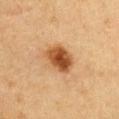This lesion was catalogued during total-body skin photography and was not selected for biopsy.
This is a cross-polarized tile.
The subject is a female about 30 years old.
Approximately 4 mm at its widest.
The lesion is on the front of the torso.
A 15 mm close-up tile from a total-body photography series done for melanoma screening.